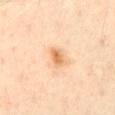| feature | finding |
|---|---|
| biopsy status | total-body-photography surveillance lesion; no biopsy |
| location | the abdomen |
| patient | male, approximately 55 years of age |
| image source | ~15 mm crop, total-body skin-cancer survey |
| illumination | cross-polarized illumination |
| lesion diameter | about 2.5 mm |